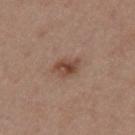<tbp_lesion>
  <biopsy_status>not biopsied; imaged during a skin examination</biopsy_status>
  <site>mid back</site>
  <patient>
    <sex>female</sex>
    <age_approx>45</age_approx>
  </patient>
  <image>
    <source>total-body photography crop</source>
    <field_of_view_mm>15</field_of_view_mm>
  </image>
  <lighting>white-light</lighting>
  <automated_metrics>
    <cielab_L>46</cielab_L>
    <cielab_a>19</cielab_a>
    <cielab_b>27</cielab_b>
    <vs_skin_darker_L>10.0</vs_skin_darker_L>
    <vs_skin_contrast_norm>8.0</vs_skin_contrast_norm>
    <border_irregularity_0_10>2.0</border_irregularity_0_10>
    <color_variation_0_10>5.0</color_variation_0_10>
    <peripheral_color_asymmetry>1.5</peripheral_color_asymmetry>
  </automated_metrics>
</tbp_lesion>